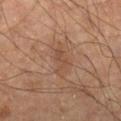{"biopsy_status": "not biopsied; imaged during a skin examination", "lighting": "cross-polarized", "image": {"source": "total-body photography crop", "field_of_view_mm": 15}, "site": "right lower leg", "lesion_size": {"long_diameter_mm_approx": 3.0}, "patient": {"sex": "male", "age_approx": 55}}